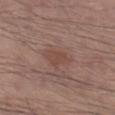The lesion was photographed on a routine skin check and not biopsied; there is no pathology result. A male subject, aged approximately 30. Approximately 3 mm at its widest. The lesion-visualizer software estimated a shape eccentricity near 0.75 and two-axis asymmetry of about 0.25. The software also gave an automated nevus-likeness rating near 0 out of 100. The lesion is on the right lower leg. This is a white-light tile. A close-up tile cropped from a whole-body skin photograph, about 15 mm across.